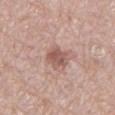Captured during whole-body skin photography for melanoma surveillance; the lesion was not biopsied.
The lesion is located on the lower back.
Cropped from a total-body skin-imaging series; the visible field is about 15 mm.
A male subject, roughly 70 years of age.